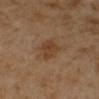workup: imaged on a skin check; not biopsied | image-analysis metrics: an area of roughly 7.5 mm², an outline eccentricity of about 0.7 (0 = round, 1 = elongated), and two-axis asymmetry of about 0.2; an average lesion color of about L≈42 a*≈18 b*≈32 (CIELAB), a lesion–skin lightness drop of about 7, and a lesion-to-skin contrast of about 6.5 (normalized; higher = more distinct); a border-irregularity rating of about 2/10, a color-variation rating of about 2.5/10, and a peripheral color-asymmetry measure near 1 | location: the right lower leg | tile lighting: cross-polarized illumination | lesion size: ~3.5 mm (longest diameter) | patient: female, approximately 50 years of age | imaging modality: ~15 mm crop, total-body skin-cancer survey.